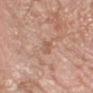Case summary:
* notes — imaged on a skin check; not biopsied
* image — 15 mm crop, total-body photography
* image-analysis metrics — a mean CIELAB color near L≈58 a*≈21 b*≈29, a lesion–skin lightness drop of about 7, and a normalized lesion–skin contrast near 5; a nevus-likeness score of about 0/100
* patient — female, aged approximately 55
* lesion size — ≈3.5 mm
* body site — the right forearm